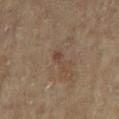Image and clinical context:
The tile uses cross-polarized illumination. The total-body-photography lesion software estimated an eccentricity of roughly 0.95 and two-axis asymmetry of about 0.35. And it measured roughly 6 lightness units darker than nearby skin and a normalized border contrast of about 5.5. And it measured a border-irregularity index near 4.5/10 and a color-variation rating of about 0/10. It also reported an automated nevus-likeness rating near 0 out of 100 and a lesion-detection confidence of about 100/100. On the right arm. A region of skin cropped from a whole-body photographic capture, roughly 15 mm wide. The patient is a female approximately 80 years of age.